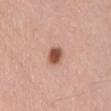Recorded during total-body skin imaging; not selected for excision or biopsy. The tile uses white-light illumination. Automated tile analysis of the lesion measured an area of roughly 4.5 mm² and two-axis asymmetry of about 0.2. The analysis additionally found a lesion–skin lightness drop of about 16 and a lesion-to-skin contrast of about 11 (normalized; higher = more distinct). The analysis additionally found a classifier nevus-likeness of about 100/100 and a detector confidence of about 100 out of 100 that the crop contains a lesion. From the mid back. A 15 mm crop from a total-body photograph taken for skin-cancer surveillance. The recorded lesion diameter is about 3 mm. A male subject, in their 60s.